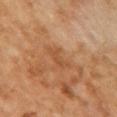Captured during whole-body skin photography for melanoma surveillance; the lesion was not biopsied.
The tile uses cross-polarized illumination.
A female patient, aged 58–62.
The lesion is on the chest.
The lesion's longest dimension is about 3 mm.
A 15 mm crop from a total-body photograph taken for skin-cancer surveillance.